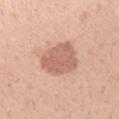Q: Was this lesion biopsied?
A: total-body-photography surveillance lesion; no biopsy
Q: How was this image acquired?
A: total-body-photography crop, ~15 mm field of view
Q: What is the anatomic site?
A: the arm
Q: Who is the patient?
A: female, in their mid-20s